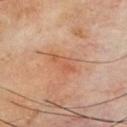Notes:
– notes — imaged on a skin check; not biopsied
– lesion size — ≈4 mm
– patient — male, aged around 70
– automated metrics — a nevus-likeness score of about 0/100 and a lesion-detection confidence of about 100/100
– anatomic site — the chest
– image source — 15 mm crop, total-body photography